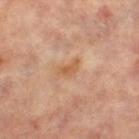Part of a total-body skin-imaging series; this lesion was reviewed on a skin check and was not flagged for biopsy.
About 2.5 mm across.
Automated tile analysis of the lesion measured an area of roughly 3 mm², an outline eccentricity of about 0.85 (0 = round, 1 = elongated), and two-axis asymmetry of about 0.45. The analysis additionally found an average lesion color of about L≈56 a*≈22 b*≈36 (CIELAB) and a normalized border contrast of about 7. The analysis additionally found a border-irregularity rating of about 4.5/10, a within-lesion color-variation index near 0/10, and a peripheral color-asymmetry measure near 0.
A female subject aged 63 to 67.
The tile uses cross-polarized illumination.
On the leg.
Cropped from a whole-body photographic skin survey; the tile spans about 15 mm.